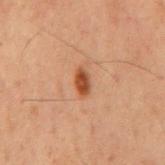biopsy_status: not biopsied; imaged during a skin examination
lighting: cross-polarized
image:
  source: total-body photography crop
  field_of_view_mm: 15
lesion_size:
  long_diameter_mm_approx: 3.0
site: back
automated_metrics:
  area_mm2_approx: 3.5
  eccentricity: 0.85
  shape_asymmetry: 0.25
  cielab_L: 37
  cielab_a: 21
  cielab_b: 30
  vs_skin_darker_L: 11.0
  vs_skin_contrast_norm: 10.0
  lesion_detection_confidence_0_100: 100
patient:
  sex: male
  age_approx: 60